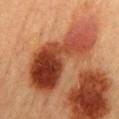No biopsy was performed on this lesion — it was imaged during a full skin examination and was not determined to be concerning.
A female subject aged 58–62.
Cropped from a whole-body photographic skin survey; the tile spans about 15 mm.
Approximately 11.5 mm at its widest.
The tile uses cross-polarized illumination.
The lesion is on the mid back.